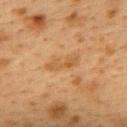| field | value |
|---|---|
| notes | catalogued during a skin exam; not biopsied |
| acquisition | total-body-photography crop, ~15 mm field of view |
| diameter | ≈4 mm |
| subject | female, approximately 40 years of age |
| location | the upper back |
| illumination | cross-polarized |
| automated metrics | a footprint of about 5 mm², an outline eccentricity of about 0.95 (0 = round, 1 = elongated), and two-axis asymmetry of about 0.25; a mean CIELAB color near L≈47 a*≈18 b*≈35, roughly 6 lightness units darker than nearby skin, and a lesion-to-skin contrast of about 5.5 (normalized; higher = more distinct); a border-irregularity index near 4/10 and a peripheral color-asymmetry measure near 1; a nevus-likeness score of about 0/100 |